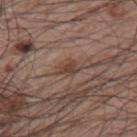Q: Is there a histopathology result?
A: no biopsy performed (imaged during a skin exam)
Q: What are the patient's age and sex?
A: male, roughly 65 years of age
Q: How was this image acquired?
A: total-body-photography crop, ~15 mm field of view
Q: How was the tile lit?
A: white-light illumination
Q: Where on the body is the lesion?
A: the left upper arm
Q: What is the lesion's diameter?
A: ~2.5 mm (longest diameter)